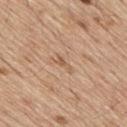follow-up — imaged on a skin check; not biopsied | body site — the mid back | subject — male, about 70 years old | image source — total-body-photography crop, ~15 mm field of view | lighting — white-light | automated lesion analysis — an outline eccentricity of about 0.95 (0 = round, 1 = elongated) and two-axis asymmetry of about 0.5; an average lesion color of about L≈58 a*≈19 b*≈33 (CIELAB), a lesion–skin lightness drop of about 8, and a normalized lesion–skin contrast near 5.5; a border-irregularity index near 5/10 and peripheral color asymmetry of about 0.